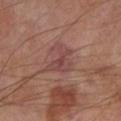Q: Was a biopsy performed?
A: no biopsy performed (imaged during a skin exam)
Q: Patient demographics?
A: male, aged approximately 70
Q: What is the imaging modality?
A: ~15 mm tile from a whole-body skin photo
Q: Automated lesion metrics?
A: a lesion area of about 7.5 mm² and an eccentricity of roughly 0.55; an average lesion color of about L≈43 a*≈22 b*≈21 (CIELAB) and a lesion–skin lightness drop of about 7; a color-variation rating of about 3/10 and radial color variation of about 1
Q: Lesion location?
A: the leg